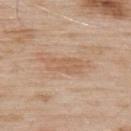Case summary:
• workup · catalogued during a skin exam; not biopsied
• automated metrics · a lesion area of about 7 mm², an eccentricity of roughly 0.9, and a symmetry-axis asymmetry near 0.15; roughly 7 lightness units darker than nearby skin and a lesion-to-skin contrast of about 5 (normalized; higher = more distinct); a border-irregularity rating of about 2.5/10, a within-lesion color-variation index near 2.5/10, and radial color variation of about 1; a classifier nevus-likeness of about 0/100 and lesion-presence confidence of about 100/100
• subject · male, aged 53–57
• acquisition · ~15 mm crop, total-body skin-cancer survey
• size · about 4.5 mm
• lighting · white-light illumination
• site · the upper back